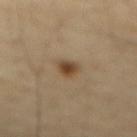Case summary:
- biopsy status · imaged on a skin check; not biopsied
- subject · male, about 65 years old
- TBP lesion metrics · a lesion area of about 4 mm², an eccentricity of roughly 0.65, and two-axis asymmetry of about 0.3; a lesion color around L≈44 a*≈15 b*≈32 in CIELAB, about 11 CIELAB-L* units darker than the surrounding skin, and a normalized border contrast of about 9
- tile lighting · cross-polarized
- location · the abdomen
- acquisition · total-body-photography crop, ~15 mm field of view